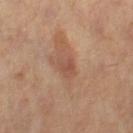Q: Is there a histopathology result?
A: total-body-photography surveillance lesion; no biopsy
Q: What lighting was used for the tile?
A: cross-polarized
Q: What is the anatomic site?
A: the right thigh
Q: Patient demographics?
A: female, aged approximately 40
Q: What kind of image is this?
A: 15 mm crop, total-body photography
Q: What is the lesion's diameter?
A: about 2.5 mm